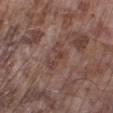Captured during whole-body skin photography for melanoma surveillance; the lesion was not biopsied.
A lesion tile, about 15 mm wide, cut from a 3D total-body photograph.
Imaged with white-light lighting.
Located on the leg.
The subject is a male aged around 75.